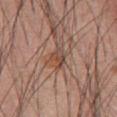Clinical impression: Recorded during total-body skin imaging; not selected for excision or biopsy. Background: A 15 mm close-up extracted from a 3D total-body photography capture. On the abdomen. A male subject approximately 60 years of age.